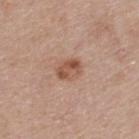{
  "biopsy_status": "not biopsied; imaged during a skin examination",
  "automated_metrics": {
    "area_mm2_approx": 6.0,
    "shape_asymmetry": 0.2,
    "cielab_L": 53,
    "cielab_a": 21,
    "cielab_b": 29,
    "vs_skin_darker_L": 10.0,
    "vs_skin_contrast_norm": 7.5,
    "border_irregularity_0_10": 2.0,
    "color_variation_0_10": 5.5,
    "nevus_likeness_0_100": 65
  },
  "lighting": "white-light",
  "image": {
    "source": "total-body photography crop",
    "field_of_view_mm": 15
  },
  "patient": {
    "sex": "male",
    "age_approx": 55
  }
}